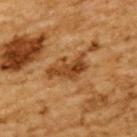Part of a total-body skin-imaging series; this lesion was reviewed on a skin check and was not flagged for biopsy.
A male subject about 85 years old or older.
Imaged with cross-polarized lighting.
The lesion is on the upper back.
Approximately 5 mm at its widest.
A 15 mm close-up extracted from a 3D total-body photography capture.